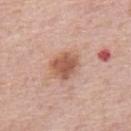Findings:
– image source — ~15 mm tile from a whole-body skin photo
– anatomic site — the back
– size — ≈3.5 mm
– illumination — white-light illumination
– subject — male, about 75 years old
– automated metrics — a border-irregularity rating of about 1.5/10 and a within-lesion color-variation index near 2.5/10